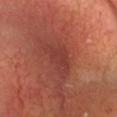Q: What kind of image is this?
A: ~15 mm crop, total-body skin-cancer survey
Q: Lesion location?
A: the head or neck
Q: Who is the patient?
A: male, in their mid-50s
Q: Lesion size?
A: about 4 mm
Q: What lighting was used for the tile?
A: cross-polarized illumination